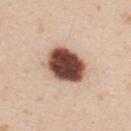The lesion was tiled from a total-body skin photograph and was not biopsied. Approximately 5.5 mm at its widest. A male subject, aged around 25. The lesion is on the upper back. The tile uses white-light illumination. A 15 mm close-up extracted from a 3D total-body photography capture.